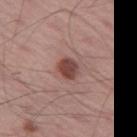biopsy_status: not biopsied; imaged during a skin examination
image:
  source: total-body photography crop
  field_of_view_mm: 15
lesion_size:
  long_diameter_mm_approx: 2.5
patient:
  sex: male
  age_approx: 55
site: left thigh
lighting: white-light